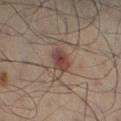No biopsy was performed on this lesion — it was imaged during a full skin examination and was not determined to be concerning.
This image is a 15 mm lesion crop taken from a total-body photograph.
The patient is a male aged approximately 50.
On the left leg.
The lesion's longest dimension is about 2.5 mm.